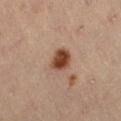Recorded during total-body skin imaging; not selected for excision or biopsy. A 15 mm crop from a total-body photograph taken for skin-cancer surveillance. Approximately 3 mm at its widest. The patient is a male in their mid- to late 50s. The lesion is located on the right thigh.